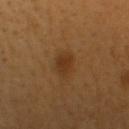Captured during whole-body skin photography for melanoma surveillance; the lesion was not biopsied. The tile uses cross-polarized illumination. A roughly 15 mm field-of-view crop from a total-body skin photograph. The lesion is located on the head or neck. The lesion-visualizer software estimated a lesion area of about 5.5 mm² and two-axis asymmetry of about 0.15. The software also gave a border-irregularity index near 1.5/10 and peripheral color asymmetry of about 0.5. And it measured a nevus-likeness score of about 100/100 and a detector confidence of about 100 out of 100 that the crop contains a lesion. Longest diameter approximately 3.5 mm. A male patient aged approximately 60.